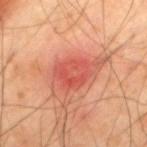Imaged during a routine full-body skin examination; the lesion was not biopsied and no histopathology is available. The patient is a male aged 38–42. A roughly 15 mm field-of-view crop from a total-body skin photograph. On the upper back. Measured at roughly 5 mm in maximum diameter. Imaged with cross-polarized lighting.